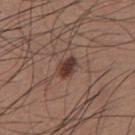workup: catalogued during a skin exam; not biopsied | subject: male, aged approximately 35 | lesion diameter: about 2.5 mm | image-analysis metrics: border irregularity of about 1.5 on a 0–10 scale and a within-lesion color-variation index near 4/10 | site: the chest | acquisition: 15 mm crop, total-body photography.